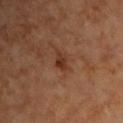Case summary:
• biopsy status: imaged on a skin check; not biopsied
• size: ≈2.5 mm
• illumination: cross-polarized
• automated metrics: an average lesion color of about L≈33 a*≈22 b*≈30 (CIELAB) and a lesion-to-skin contrast of about 7.5 (normalized; higher = more distinct); border irregularity of about 3.5 on a 0–10 scale and internal color variation of about 3.5 on a 0–10 scale; a nevus-likeness score of about 15/100 and a detector confidence of about 100 out of 100 that the crop contains a lesion
• subject: male, about 65 years old
• image: ~15 mm tile from a whole-body skin photo
• anatomic site: the chest